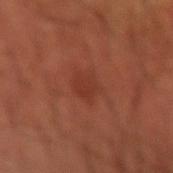  biopsy_status: not biopsied; imaged during a skin examination
  image:
    source: total-body photography crop
    field_of_view_mm: 15
  site: right forearm
  automated_metrics:
    cielab_L: 35
    cielab_a: 27
    cielab_b: 30
    vs_skin_darker_L: 5.0
    vs_skin_contrast_norm: 5.0
    border_irregularity_0_10: 2.5
    color_variation_0_10: 1.5
    nevus_likeness_0_100: 0
    lesion_detection_confidence_0_100: 100
  patient:
    sex: male
    age_approx: 55
  lighting: cross-polarized
  lesion_size:
    long_diameter_mm_approx: 3.0